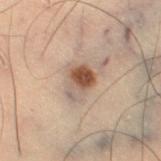Imaged with cross-polarized lighting.
The lesion-visualizer software estimated a footprint of about 7 mm², an outline eccentricity of about 0.7 (0 = round, 1 = elongated), and two-axis asymmetry of about 0.3. It also reported a mean CIELAB color near L≈42 a*≈15 b*≈23, roughly 12 lightness units darker than nearby skin, and a lesion-to-skin contrast of about 9.5 (normalized; higher = more distinct).
Measured at roughly 3.5 mm in maximum diameter.
A region of skin cropped from a whole-body photographic capture, roughly 15 mm wide.
Located on the right thigh.
A male subject aged 53–57.